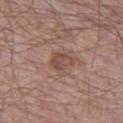On the left thigh. Imaged with white-light lighting. A 15 mm crop from a total-body photograph taken for skin-cancer surveillance. A male subject, approximately 65 years of age. Approximately 2.5 mm at its widest.